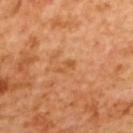This lesion was catalogued during total-body skin photography and was not selected for biopsy. A 15 mm close-up tile from a total-body photography series done for melanoma screening. The patient is a female approximately 55 years of age. This is a cross-polarized tile. Automated image analysis of the tile measured a footprint of about 2 mm² and two-axis asymmetry of about 0.5. The analysis additionally found a mean CIELAB color near L≈57 a*≈27 b*≈44, roughly 7 lightness units darker than nearby skin, and a normalized border contrast of about 5.5. And it measured internal color variation of about 0 on a 0–10 scale and radial color variation of about 0. And it measured a nevus-likeness score of about 0/100. The lesion is on the upper back.